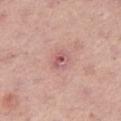Imaged during a routine full-body skin examination; the lesion was not biopsied and no histopathology is available. The subject is a male roughly 50 years of age. A close-up tile cropped from a whole-body skin photograph, about 15 mm across. Located on the leg.